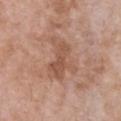The lesion was tiled from a total-body skin photograph and was not biopsied.